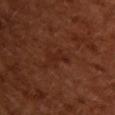follow-up: no biopsy performed (imaged during a skin exam) | automated lesion analysis: a lesion area of about 3 mm², an eccentricity of roughly 0.9, and two-axis asymmetry of about 0.55; a mean CIELAB color near L≈25 a*≈24 b*≈28 and about 5 CIELAB-L* units darker than the surrounding skin; a border-irregularity rating of about 6/10, a within-lesion color-variation index near 0/10, and a peripheral color-asymmetry measure near 0 | patient: female, aged 53 to 57 | body site: the upper back | image: ~15 mm crop, total-body skin-cancer survey | lesion size: ~3 mm (longest diameter) | illumination: cross-polarized.